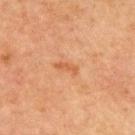Impression:
Recorded during total-body skin imaging; not selected for excision or biopsy.
Image and clinical context:
An algorithmic analysis of the crop reported a lesion color around L≈47 a*≈22 b*≈34 in CIELAB and roughly 6 lightness units darker than nearby skin. A 15 mm close-up tile from a total-body photography series done for melanoma screening. The tile uses cross-polarized illumination. A male subject in their mid- to late 60s. Approximately 3 mm at its widest. On the chest.